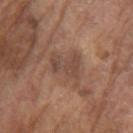Impression: The lesion was photographed on a routine skin check and not biopsied; there is no pathology result. Image and clinical context: A 15 mm close-up extracted from a 3D total-body photography capture. Located on the left upper arm. The subject is a male approximately 80 years of age. Approximately 4.5 mm at its widest.